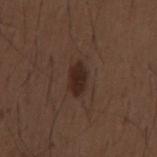Assessment: Recorded during total-body skin imaging; not selected for excision or biopsy. Context: The total-body-photography lesion software estimated a mean CIELAB color near L≈26 a*≈16 b*≈22, roughly 9 lightness units darker than nearby skin, and a normalized border contrast of about 9.5. It also reported a border-irregularity index near 2/10, a color-variation rating of about 2.5/10, and peripheral color asymmetry of about 1. A male patient, aged approximately 50. On the mid back. A 15 mm close-up extracted from a 3D total-body photography capture.